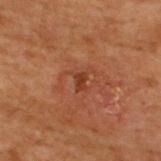| field | value |
|---|---|
| size | about 1.5 mm |
| image source | ~15 mm crop, total-body skin-cancer survey |
| site | the upper back |
| subject | male, aged approximately 70 |